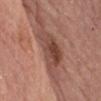Assessment:
Imaged during a routine full-body skin examination; the lesion was not biopsied and no histopathology is available.
Background:
Measured at roughly 6 mm in maximum diameter. A lesion tile, about 15 mm wide, cut from a 3D total-body photograph. The patient is a male aged 58 to 62. Located on the front of the torso.